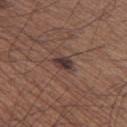Assessment:
Recorded during total-body skin imaging; not selected for excision or biopsy.
Background:
The lesion's longest dimension is about 3 mm. Captured under white-light illumination. A male subject, aged around 65. The lesion is located on the left thigh. The total-body-photography lesion software estimated a footprint of about 4 mm². And it measured a lesion color around L≈35 a*≈16 b*≈18 in CIELAB, about 11 CIELAB-L* units darker than the surrounding skin, and a normalized lesion–skin contrast near 10.5. The software also gave a lesion-detection confidence of about 100/100. A close-up tile cropped from a whole-body skin photograph, about 15 mm across.